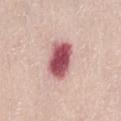Clinical impression:
Part of a total-body skin-imaging series; this lesion was reviewed on a skin check and was not flagged for biopsy.
Image and clinical context:
A close-up tile cropped from a whole-body skin photograph, about 15 mm across. Approximately 4.5 mm at its widest. A female patient approximately 65 years of age. This is a white-light tile. The lesion is located on the back.